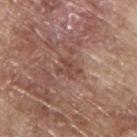Imaged during a routine full-body skin examination; the lesion was not biopsied and no histopathology is available. An algorithmic analysis of the crop reported a mean CIELAB color near L≈46 a*≈20 b*≈25, roughly 8 lightness units darker than nearby skin, and a normalized lesion–skin contrast near 6. And it measured a border-irregularity index near 4/10 and a peripheral color-asymmetry measure near 1. And it measured a classifier nevus-likeness of about 0/100 and lesion-presence confidence of about 95/100. A roughly 15 mm field-of-view crop from a total-body skin photograph. The lesion is on the upper back. A male patient, about 65 years old. Captured under white-light illumination. Longest diameter approximately 3 mm.